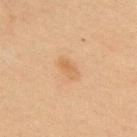The lesion was tiled from a total-body skin photograph and was not biopsied. A male subject aged around 60. The tile uses cross-polarized illumination. This image is a 15 mm lesion crop taken from a total-body photograph. The lesion-visualizer software estimated a border-irregularity rating of about 2.5/10, a within-lesion color-variation index near 3/10, and a peripheral color-asymmetry measure near 1. And it measured a nevus-likeness score of about 65/100 and a lesion-detection confidence of about 100/100. The lesion is located on the upper back.